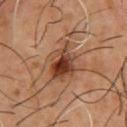workup = catalogued during a skin exam; not biopsied
location = the chest
image source = ~15 mm crop, total-body skin-cancer survey
lesion diameter = about 5 mm
TBP lesion metrics = a within-lesion color-variation index near 9/10 and a peripheral color-asymmetry measure near 2.5; a nevus-likeness score of about 85/100 and a lesion-detection confidence of about 100/100
subject = male, aged around 55
tile lighting = cross-polarized illumination